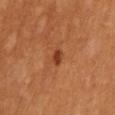This lesion was catalogued during total-body skin photography and was not selected for biopsy.